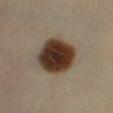The lesion was photographed on a routine skin check and not biopsied; there is no pathology result.
Imaged with cross-polarized lighting.
Located on the right lower leg.
Measured at roughly 5 mm in maximum diameter.
A female subject in their mid-50s.
A lesion tile, about 15 mm wide, cut from a 3D total-body photograph.